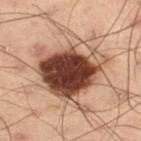Part of a total-body skin-imaging series; this lesion was reviewed on a skin check and was not flagged for biopsy. The tile uses cross-polarized illumination. From the right thigh. The recorded lesion diameter is about 7.5 mm. Automated tile analysis of the lesion measured a mean CIELAB color near L≈36 a*≈18 b*≈23, a lesion–skin lightness drop of about 19, and a lesion-to-skin contrast of about 15 (normalized; higher = more distinct). The software also gave a classifier nevus-likeness of about 85/100 and a detector confidence of about 100 out of 100 that the crop contains a lesion. A male subject, aged 53 to 57. Cropped from a total-body skin-imaging series; the visible field is about 15 mm.